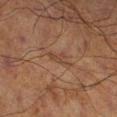follow-up: total-body-photography surveillance lesion; no biopsy | patient: male, roughly 70 years of age | location: the right lower leg | size: ≈2.5 mm | image: ~15 mm tile from a whole-body skin photo.